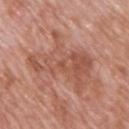Q: Was a biopsy performed?
A: no biopsy performed (imaged during a skin exam)
Q: What did automated image analysis measure?
A: an area of roughly 25 mm², an eccentricity of roughly 0.85, and a shape-asymmetry score of about 0.5 (0 = symmetric); a lesion color around L≈53 a*≈24 b*≈29 in CIELAB, about 8 CIELAB-L* units darker than the surrounding skin, and a normalized lesion–skin contrast near 6; border irregularity of about 8.5 on a 0–10 scale, internal color variation of about 5 on a 0–10 scale, and a peripheral color-asymmetry measure near 2
Q: What is the lesion's diameter?
A: ~8.5 mm (longest diameter)
Q: Patient demographics?
A: male, aged 68 to 72
Q: Where on the body is the lesion?
A: the upper back
Q: How was this image acquired?
A: ~15 mm crop, total-body skin-cancer survey